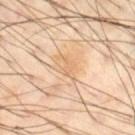biopsy status = no biopsy performed (imaged during a skin exam); diameter = about 3 mm; illumination = cross-polarized illumination; acquisition = 15 mm crop, total-body photography; site = the leg; subject = male, in their 50s.